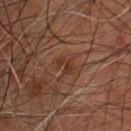Q: Is there a histopathology result?
A: catalogued during a skin exam; not biopsied
Q: What kind of image is this?
A: ~15 mm crop, total-body skin-cancer survey
Q: Lesion size?
A: about 2.5 mm
Q: Lesion location?
A: the chest
Q: Patient demographics?
A: male, about 55 years old